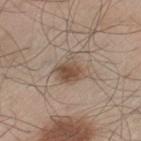Impression:
Recorded during total-body skin imaging; not selected for excision or biopsy.
Acquisition and patient details:
Automated image analysis of the tile measured a lesion area of about 9 mm² and a shape eccentricity near 0.6. The analysis additionally found a lesion color around L≈51 a*≈14 b*≈26 in CIELAB, a lesion–skin lightness drop of about 10, and a normalized border contrast of about 7.5. The analysis additionally found a detector confidence of about 100 out of 100 that the crop contains a lesion. Measured at roughly 4 mm in maximum diameter. The subject is a male roughly 60 years of age. The lesion is located on the leg. A region of skin cropped from a whole-body photographic capture, roughly 15 mm wide. Captured under white-light illumination.